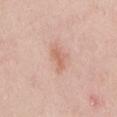Impression: Part of a total-body skin-imaging series; this lesion was reviewed on a skin check and was not flagged for biopsy. Background: From the chest. A male patient in their 50s. The lesion-visualizer software estimated a footprint of about 3.5 mm² and two-axis asymmetry of about 0.3. And it measured a lesion-detection confidence of about 100/100. A 15 mm crop from a total-body photograph taken for skin-cancer surveillance. Imaged with white-light lighting. The recorded lesion diameter is about 3 mm.